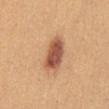Assessment:
The lesion was tiled from a total-body skin photograph and was not biopsied.
Background:
A female patient, roughly 25 years of age. About 4.5 mm across. On the abdomen. A lesion tile, about 15 mm wide, cut from a 3D total-body photograph. The tile uses white-light illumination.